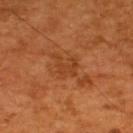Clinical impression: Imaged during a routine full-body skin examination; the lesion was not biopsied and no histopathology is available. Clinical summary: Longest diameter approximately 3 mm. The subject is a male aged around 55. This is a cross-polarized tile. From the upper back. A lesion tile, about 15 mm wide, cut from a 3D total-body photograph.